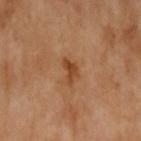workup = imaged on a skin check; not biopsied.